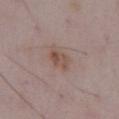Clinical impression: The lesion was photographed on a routine skin check and not biopsied; there is no pathology result. Context: An algorithmic analysis of the crop reported a footprint of about 6 mm² and two-axis asymmetry of about 0.25. The software also gave a lesion color around L≈51 a*≈17 b*≈24 in CIELAB, roughly 8 lightness units darker than nearby skin, and a normalized lesion–skin contrast near 7. The software also gave a border-irregularity rating of about 2.5/10, a color-variation rating of about 5/10, and radial color variation of about 1.5. And it measured a classifier nevus-likeness of about 65/100 and a lesion-detection confidence of about 100/100. A 15 mm crop from a total-body photograph taken for skin-cancer surveillance. The tile uses white-light illumination. Located on the abdomen. A male patient aged 48 to 52.